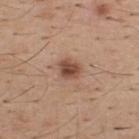Q: Automated lesion metrics?
A: an area of roughly 5 mm², a shape eccentricity near 0.6, and a symmetry-axis asymmetry near 0.25; a mean CIELAB color near L≈48 a*≈21 b*≈28, roughly 13 lightness units darker than nearby skin, and a normalized border contrast of about 9.5; a border-irregularity rating of about 2/10, a within-lesion color-variation index near 4.5/10, and radial color variation of about 1.5; a nevus-likeness score of about 90/100 and lesion-presence confidence of about 100/100
Q: Patient demographics?
A: male, approximately 40 years of age
Q: What is the lesion's diameter?
A: ≈2.5 mm
Q: Where on the body is the lesion?
A: the back
Q: What is the imaging modality?
A: 15 mm crop, total-body photography